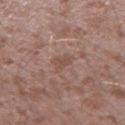notes: catalogued during a skin exam; not biopsied
lighting: white-light illumination
automated lesion analysis: an area of roughly 4 mm², an outline eccentricity of about 0.6 (0 = round, 1 = elongated), and a shape-asymmetry score of about 0.35 (0 = symmetric); a lesion color around L≈50 a*≈19 b*≈24 in CIELAB, about 6 CIELAB-L* units darker than the surrounding skin, and a lesion-to-skin contrast of about 5 (normalized; higher = more distinct); a classifier nevus-likeness of about 0/100 and lesion-presence confidence of about 95/100
acquisition: 15 mm crop, total-body photography
site: the leg
patient: male, aged around 45
diameter: ~2.5 mm (longest diameter)